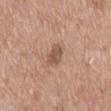Clinical impression:
No biopsy was performed on this lesion — it was imaged during a full skin examination and was not determined to be concerning.
Image and clinical context:
The patient is a male aged approximately 60. This is a white-light tile. Located on the back. The total-body-photography lesion software estimated an eccentricity of roughly 0.75 and two-axis asymmetry of about 0.2. And it measured a within-lesion color-variation index near 2/10 and peripheral color asymmetry of about 0.5. The analysis additionally found a lesion-detection confidence of about 100/100. A 15 mm crop from a total-body photograph taken for skin-cancer surveillance.